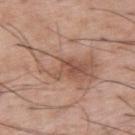Notes:
- notes — total-body-photography surveillance lesion; no biopsy
- site — the arm
- image source — ~15 mm tile from a whole-body skin photo
- patient — male, aged 53 to 57